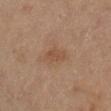Imaged during a routine full-body skin examination; the lesion was not biopsied and no histopathology is available. Cropped from a total-body skin-imaging series; the visible field is about 15 mm. Imaged with cross-polarized lighting. The lesion is located on the right lower leg. A female subject aged around 55. The total-body-photography lesion software estimated an average lesion color of about L≈41 a*≈16 b*≈27 (CIELAB), a lesion–skin lightness drop of about 6, and a normalized border contrast of about 5.5. It also reported a classifier nevus-likeness of about 10/100 and a lesion-detection confidence of about 100/100. Measured at roughly 3 mm in maximum diameter.